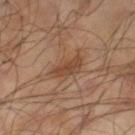Background: The lesion is located on the leg. A male patient, in their mid- to late 60s. A 15 mm close-up extracted from a 3D total-body photography capture.